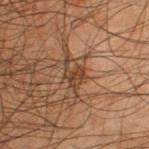- diameter: about 3.5 mm
- image-analysis metrics: a footprint of about 5 mm², an outline eccentricity of about 0.75 (0 = round, 1 = elongated), and a symmetry-axis asymmetry near 0.45; an average lesion color of about L≈34 a*≈16 b*≈26 (CIELAB), roughly 8 lightness units darker than nearby skin, and a normalized border contrast of about 7.5; a border-irregularity index near 6.5/10 and radial color variation of about 1; a lesion-detection confidence of about 60/100
- image: ~15 mm crop, total-body skin-cancer survey
- location: the left thigh
- subject: male, aged around 65